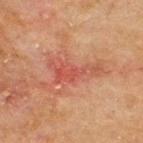Acquisition and patient details: On the upper back. A male patient, in their 70s. A close-up tile cropped from a whole-body skin photograph, about 15 mm across.